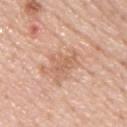notes: total-body-photography surveillance lesion; no biopsy | patient: male, about 45 years old | image: ~15 mm tile from a whole-body skin photo | anatomic site: the upper back.